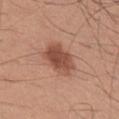{"biopsy_status": "not biopsied; imaged during a skin examination", "lesion_size": {"long_diameter_mm_approx": 5.0}, "patient": {"sex": "male", "age_approx": 30}, "image": {"source": "total-body photography crop", "field_of_view_mm": 15}, "automated_metrics": {"border_irregularity_0_10": 2.0, "color_variation_0_10": 4.5, "peripheral_color_asymmetry": 1.5, "nevus_likeness_0_100": 95}, "site": "chest", "lighting": "white-light"}